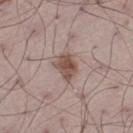notes: no biopsy performed (imaged during a skin exam) | image: total-body-photography crop, ~15 mm field of view | patient: male, about 70 years old | lesion diameter: about 3.5 mm | image-analysis metrics: border irregularity of about 2.5 on a 0–10 scale, a within-lesion color-variation index near 3.5/10, and radial color variation of about 1 | tile lighting: white-light illumination | site: the left thigh.